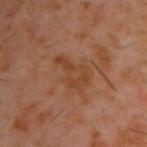biopsy status: no biopsy performed (imaged during a skin exam)
acquisition: ~15 mm tile from a whole-body skin photo
illumination: cross-polarized
anatomic site: the upper back
patient: male, about 60 years old
size: ≈4.5 mm
automated lesion analysis: an area of roughly 8.5 mm², an outline eccentricity of about 0.85 (0 = round, 1 = elongated), and a symmetry-axis asymmetry near 0.5; an automated nevus-likeness rating near 0 out of 100 and a lesion-detection confidence of about 100/100